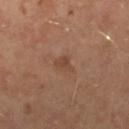Captured during whole-body skin photography for melanoma surveillance; the lesion was not biopsied.
The total-body-photography lesion software estimated a lesion area of about 3.5 mm² and a shape eccentricity near 0.8. It also reported a lesion color around L≈44 a*≈20 b*≈29 in CIELAB, about 6 CIELAB-L* units darker than the surrounding skin, and a normalized border contrast of about 5.5. It also reported a border-irregularity index near 3.5/10, internal color variation of about 1.5 on a 0–10 scale, and radial color variation of about 0.5. It also reported an automated nevus-likeness rating near 0 out of 100.
A 15 mm crop from a total-body photograph taken for skin-cancer surveillance.
Measured at roughly 2.5 mm in maximum diameter.
A male subject, aged approximately 50.
On the left leg.